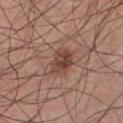biopsy status = catalogued during a skin exam; not biopsied | diameter = ≈3.5 mm | illumination = white-light illumination | anatomic site = the chest | acquisition = ~15 mm tile from a whole-body skin photo | subject = male, aged 28 to 32.